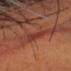Clinical impression:
No biopsy was performed on this lesion — it was imaged during a full skin examination and was not determined to be concerning.
Background:
The patient is a male approximately 60 years of age. The lesion is located on the head or neck. The tile uses cross-polarized illumination. A 15 mm crop from a total-body photograph taken for skin-cancer surveillance. Approximately 3 mm at its widest.